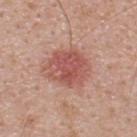Assessment:
The lesion was photographed on a routine skin check and not biopsied; there is no pathology result.
Context:
Longest diameter approximately 5 mm. Automated image analysis of the tile measured a color-variation rating of about 4/10 and a peripheral color-asymmetry measure near 1.5. The analysis additionally found a detector confidence of about 100 out of 100 that the crop contains a lesion. The subject is a male roughly 40 years of age. Located on the upper back. This is a white-light tile. A lesion tile, about 15 mm wide, cut from a 3D total-body photograph.